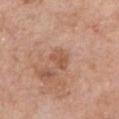This lesion was catalogued during total-body skin photography and was not selected for biopsy.
A male patient, aged around 60.
Cropped from a total-body skin-imaging series; the visible field is about 15 mm.
This is a white-light tile.
The lesion is on the chest.
Longest diameter approximately 3 mm.